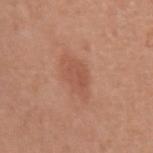  biopsy_status: not biopsied; imaged during a skin examination
  image:
    source: total-body photography crop
    field_of_view_mm: 15
  lesion_size:
    long_diameter_mm_approx: 4.5
  site: arm
  patient:
    sex: female
    age_approx: 50
  lighting: white-light